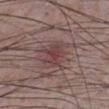{"biopsy_status": "not biopsied; imaged during a skin examination", "image": {"source": "total-body photography crop", "field_of_view_mm": 15}, "lesion_size": {"long_diameter_mm_approx": 3.0}, "lighting": "white-light", "automated_metrics": {"area_mm2_approx": 7.0, "eccentricity": 0.3, "shape_asymmetry": 0.35, "nevus_likeness_0_100": 100, "lesion_detection_confidence_0_100": 100}, "site": "left lower leg", "patient": {"sex": "male", "age_approx": 75}}